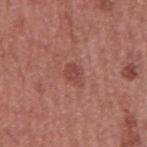Imaged during a routine full-body skin examination; the lesion was not biopsied and no histopathology is available.
An algorithmic analysis of the crop reported an area of roughly 4 mm², an outline eccentricity of about 0.7 (0 = round, 1 = elongated), and two-axis asymmetry of about 0.25.
The lesion is on the arm.
This is a white-light tile.
A lesion tile, about 15 mm wide, cut from a 3D total-body photograph.
A male subject in their mid-40s.
Longest diameter approximately 2.5 mm.